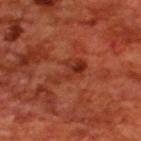The lesion was tiled from a total-body skin photograph and was not biopsied.
The patient is a male aged 68–72.
Automated tile analysis of the lesion measured an outline eccentricity of about 0.9 (0 = round, 1 = elongated) and two-axis asymmetry of about 0.55. And it measured a lesion–skin lightness drop of about 7 and a normalized lesion–skin contrast near 6.5. And it measured an automated nevus-likeness rating near 0 out of 100 and a detector confidence of about 100 out of 100 that the crop contains a lesion.
Imaged with cross-polarized lighting.
A 15 mm close-up extracted from a 3D total-body photography capture.
The lesion is on the upper back.
Measured at roughly 4.5 mm in maximum diameter.